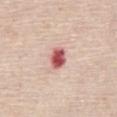This lesion was catalogued during total-body skin photography and was not selected for biopsy. Captured under white-light illumination. Located on the front of the torso. Automated tile analysis of the lesion measured a symmetry-axis asymmetry near 0.15. It also reported an average lesion color of about L≈57 a*≈32 b*≈25 (CIELAB), roughly 19 lightness units darker than nearby skin, and a normalized lesion–skin contrast near 11.5. And it measured a border-irregularity rating of about 1.5/10 and a color-variation rating of about 3.5/10. The software also gave a classifier nevus-likeness of about 0/100. A lesion tile, about 15 mm wide, cut from a 3D total-body photograph. A female subject, aged 68 to 72.